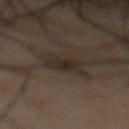Imaged during a routine full-body skin examination; the lesion was not biopsied and no histopathology is available. The lesion-visualizer software estimated a lesion area of about 6 mm² and two-axis asymmetry of about 0.2. And it measured an automated nevus-likeness rating near 0 out of 100 and a lesion-detection confidence of about 90/100. The subject is a male aged approximately 50. A lesion tile, about 15 mm wide, cut from a 3D total-body photograph. The lesion is on the front of the torso.